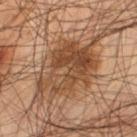workup: catalogued during a skin exam; not biopsied
location: the left thigh
image: total-body-photography crop, ~15 mm field of view
automated metrics: an area of roughly 23 mm², an eccentricity of roughly 0.75, and a shape-asymmetry score of about 0.35 (0 = symmetric); a color-variation rating of about 7.5/10 and peripheral color asymmetry of about 3
size: ~7 mm (longest diameter)
patient: male, roughly 55 years of age